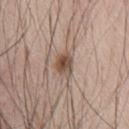Impression: Part of a total-body skin-imaging series; this lesion was reviewed on a skin check and was not flagged for biopsy. Context: The lesion is on the chest. Measured at roughly 3 mm in maximum diameter. Imaged with white-light lighting. The patient is a male in their mid- to late 40s. A 15 mm close-up extracted from a 3D total-body photography capture.